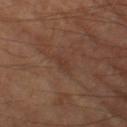Clinical impression: The lesion was photographed on a routine skin check and not biopsied; there is no pathology result. Image and clinical context: The tile uses cross-polarized illumination. A region of skin cropped from a whole-body photographic capture, roughly 15 mm wide. The lesion is on the leg. Automated image analysis of the tile measured roughly 5 lightness units darker than nearby skin and a lesion-to-skin contrast of about 4.5 (normalized; higher = more distinct). It also reported a nevus-likeness score of about 0/100. A male subject, aged around 65. Approximately 2.5 mm at its widest.